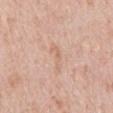workup: imaged on a skin check; not biopsied | image source: ~15 mm crop, total-body skin-cancer survey | patient: male, aged 58 to 62 | image-analysis metrics: an area of roughly 3 mm²; roughly 6 lightness units darker than nearby skin and a normalized lesion–skin contrast near 4.5; a peripheral color-asymmetry measure near 0; an automated nevus-likeness rating near 0 out of 100 and a detector confidence of about 85 out of 100 that the crop contains a lesion | lesion diameter: ~4 mm (longest diameter) | body site: the mid back | tile lighting: white-light.